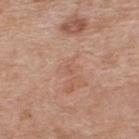Captured during whole-body skin photography for melanoma surveillance; the lesion was not biopsied. Cropped from a whole-body photographic skin survey; the tile spans about 15 mm. The lesion is on the upper back. A female patient, approximately 40 years of age.